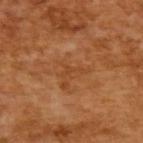follow-up: no biopsy performed (imaged during a skin exam)
image: ~15 mm crop, total-body skin-cancer survey
patient: male, approximately 65 years of age
image-analysis metrics: a footprint of about 2.5 mm², a shape eccentricity near 0.9, and a symmetry-axis asymmetry near 0.55; border irregularity of about 7 on a 0–10 scale
tile lighting: cross-polarized illumination
diameter: ~3 mm (longest diameter)